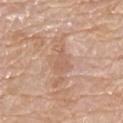<case>
  <site>upper back</site>
  <patient>
    <sex>male</sex>
    <age_approx>80</age_approx>
  </patient>
  <automated_metrics>
    <area_mm2_approx>3.5</area_mm2_approx>
    <eccentricity>0.8</eccentricity>
    <shape_asymmetry>0.4</shape_asymmetry>
  </automated_metrics>
  <lighting>white-light</lighting>
  <image>
    <source>total-body photography crop</source>
    <field_of_view_mm>15</field_of_view_mm>
  </image>
  <lesion_size>
    <long_diameter_mm_approx>2.5</long_diameter_mm_approx>
  </lesion_size>
</case>